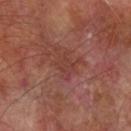Recorded during total-body skin imaging; not selected for excision or biopsy. The total-body-photography lesion software estimated a footprint of about 5 mm² and an eccentricity of roughly 0.7. The software also gave a lesion color around L≈38 a*≈24 b*≈24 in CIELAB, a lesion–skin lightness drop of about 5, and a normalized border contrast of about 4.5. A region of skin cropped from a whole-body photographic capture, roughly 15 mm wide. A male subject about 70 years old. Located on the arm. Captured under cross-polarized illumination.